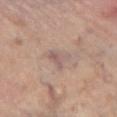patient:
  sex: male
  age_approx: 70
site: left lower leg
image:
  source: total-body photography crop
  field_of_view_mm: 15
automated_metrics:
  cielab_L: 55
  cielab_a: 16
  cielab_b: 20
  vs_skin_darker_L: 7.0
  vs_skin_contrast_norm: 5.5
  border_irregularity_0_10: 4.5
  color_variation_0_10: 2.0
  peripheral_color_asymmetry: 0.5
lesion_size:
  long_diameter_mm_approx: 2.5